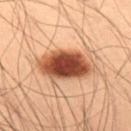Notes:
* site: the left thigh
* diameter: about 6.5 mm
* tile lighting: cross-polarized
* patient: male, roughly 55 years of age
* automated lesion analysis: a normalized border contrast of about 14.5; a detector confidence of about 100 out of 100 that the crop contains a lesion
* image source: ~15 mm crop, total-body skin-cancer survey